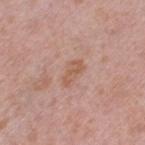Recorded during total-body skin imaging; not selected for excision or biopsy.